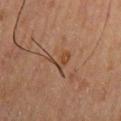Acquisition and patient details:
A male subject, approximately 70 years of age. About 3 mm across. From the mid back. Imaged with cross-polarized lighting. An algorithmic analysis of the crop reported an area of roughly 4 mm², an eccentricity of roughly 0.6, and two-axis asymmetry of about 0.7. And it measured roughly 6 lightness units darker than nearby skin and a lesion-to-skin contrast of about 6 (normalized; higher = more distinct). And it measured border irregularity of about 7.5 on a 0–10 scale, a color-variation rating of about 1.5/10, and peripheral color asymmetry of about 0.5. Cropped from a total-body skin-imaging series; the visible field is about 15 mm.